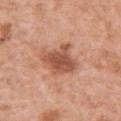Findings:
* biopsy status: catalogued during a skin exam; not biopsied
* location: the chest
* image source: 15 mm crop, total-body photography
* patient: female, aged approximately 50